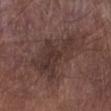Clinical impression: This lesion was catalogued during total-body skin photography and was not selected for biopsy. Clinical summary: The recorded lesion diameter is about 7.5 mm. A male subject, roughly 55 years of age. A region of skin cropped from a whole-body photographic capture, roughly 15 mm wide. An algorithmic analysis of the crop reported a classifier nevus-likeness of about 0/100. The lesion is on the right lower leg.